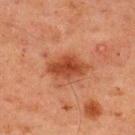follow-up: catalogued during a skin exam; not biopsied | acquisition: total-body-photography crop, ~15 mm field of view | location: the upper back | diameter: ≈4.5 mm | subject: male, roughly 60 years of age.